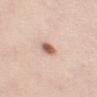The lesion was tiled from a total-body skin photograph and was not biopsied. The subject is a male aged 23–27. Cropped from a whole-body photographic skin survey; the tile spans about 15 mm. About 2.5 mm across. Captured under white-light illumination.